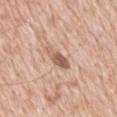Imaged during a routine full-body skin examination; the lesion was not biopsied and no histopathology is available. Cropped from a whole-body photographic skin survey; the tile spans about 15 mm. An algorithmic analysis of the crop reported an area of roughly 5 mm² and a shape-asymmetry score of about 0.25 (0 = symmetric). The software also gave a mean CIELAB color near L≈59 a*≈21 b*≈30, about 13 CIELAB-L* units darker than the surrounding skin, and a lesion-to-skin contrast of about 8 (normalized; higher = more distinct). From the mid back. A male subject approximately 50 years of age. Longest diameter approximately 4.5 mm. Captured under white-light illumination.